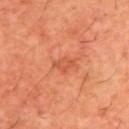Clinical impression:
Recorded during total-body skin imaging; not selected for excision or biopsy.
Clinical summary:
A male subject, roughly 60 years of age. The recorded lesion diameter is about 3 mm. On the upper back. An algorithmic analysis of the crop reported a nevus-likeness score of about 5/100. A lesion tile, about 15 mm wide, cut from a 3D total-body photograph.